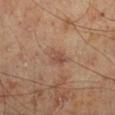This lesion was catalogued during total-body skin photography and was not selected for biopsy.
The lesion is located on the right lower leg.
Approximately 2.5 mm at its widest.
Cropped from a whole-body photographic skin survey; the tile spans about 15 mm.
A male patient roughly 70 years of age.
The tile uses cross-polarized illumination.